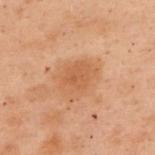workup = no biopsy performed (imaged during a skin exam) | site = the upper back | imaging modality = 15 mm crop, total-body photography | subject = female, aged 53–57.